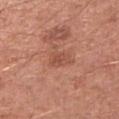Impression: Imaged during a routine full-body skin examination; the lesion was not biopsied and no histopathology is available. Acquisition and patient details: A male patient, about 55 years old. A 15 mm crop from a total-body photograph taken for skin-cancer surveillance. The lesion is located on the left upper arm. Imaged with white-light lighting.